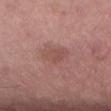A close-up tile cropped from a whole-body skin photograph, about 15 mm across. The lesion-visualizer software estimated a lesion color around L≈51 a*≈22 b*≈24 in CIELAB and a normalized border contrast of about 5. The software also gave a nevus-likeness score of about 0/100 and a detector confidence of about 100 out of 100 that the crop contains a lesion. The lesion's longest dimension is about 3.5 mm. The lesion is located on the lower back. A male subject, approximately 55 years of age.